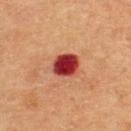Assessment:
The lesion was tiled from a total-body skin photograph and was not biopsied.
Clinical summary:
The recorded lesion diameter is about 3.5 mm. The tile uses cross-polarized illumination. Cropped from a total-body skin-imaging series; the visible field is about 15 mm. The total-body-photography lesion software estimated a lesion area of about 9 mm², an eccentricity of roughly 0.45, and a symmetry-axis asymmetry near 0.2. And it measured an average lesion color of about L≈37 a*≈35 b*≈28 (CIELAB) and a normalized lesion–skin contrast near 14. The software also gave a classifier nevus-likeness of about 0/100. The subject is a male aged 58 to 62. From the upper back.